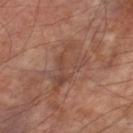| key | value |
|---|---|
| biopsy status | total-body-photography surveillance lesion; no biopsy |
| image source | total-body-photography crop, ~15 mm field of view |
| lighting | cross-polarized |
| body site | the left thigh |
| lesion size | ~6.5 mm (longest diameter) |
| patient | male, roughly 70 years of age |